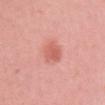Part of a total-body skin-imaging series; this lesion was reviewed on a skin check and was not flagged for biopsy.
A female patient aged around 15.
On the chest.
A roughly 15 mm field-of-view crop from a total-body skin photograph.